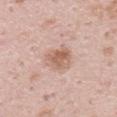Q: Was this lesion biopsied?
A: total-body-photography surveillance lesion; no biopsy
Q: Lesion location?
A: the upper back
Q: Patient demographics?
A: male, in their mid-30s
Q: How was this image acquired?
A: 15 mm crop, total-body photography
Q: Automated lesion metrics?
A: a border-irregularity rating of about 2/10, a color-variation rating of about 4/10, and peripheral color asymmetry of about 1.5; a classifier nevus-likeness of about 60/100 and a lesion-detection confidence of about 100/100
Q: How was the tile lit?
A: white-light illumination
Q: What is the lesion's diameter?
A: ~4 mm (longest diameter)